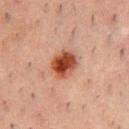From the chest.
Imaged with cross-polarized lighting.
A male patient, aged 48 to 52.
A 15 mm close-up extracted from a 3D total-body photography capture.
The lesion's longest dimension is about 3.5 mm.